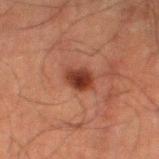Recorded during total-body skin imaging; not selected for excision or biopsy. Captured under cross-polarized illumination. A 15 mm close-up extracted from a 3D total-body photography capture. About 2.5 mm across. The lesion-visualizer software estimated a shape eccentricity near 0.65 and a symmetry-axis asymmetry near 0.2. The software also gave a mean CIELAB color near L≈29 a*≈22 b*≈25. And it measured a border-irregularity index near 1.5/10, a within-lesion color-variation index near 3.5/10, and peripheral color asymmetry of about 1. On the left thigh. The subject is a male aged approximately 50.